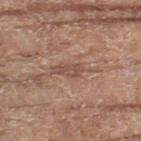Recorded during total-body skin imaging; not selected for excision or biopsy.
The lesion is on the left thigh.
Cropped from a whole-body photographic skin survey; the tile spans about 15 mm.
The patient is a female aged around 75.
The recorded lesion diameter is about 2.5 mm.
Imaged with white-light lighting.